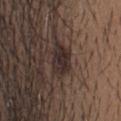Assessment: Captured during whole-body skin photography for melanoma surveillance; the lesion was not biopsied. Background: Imaged with white-light lighting. The lesion is on the head or neck. An algorithmic analysis of the crop reported an average lesion color of about L≈28 a*≈12 b*≈16 (CIELAB), about 9 CIELAB-L* units darker than the surrounding skin, and a normalized border contrast of about 10.5. This image is a 15 mm lesion crop taken from a total-body photograph. A male patient roughly 25 years of age. The recorded lesion diameter is about 4.5 mm.